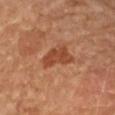Clinical impression:
Captured during whole-body skin photography for melanoma surveillance; the lesion was not biopsied.
Background:
An algorithmic analysis of the crop reported a border-irregularity rating of about 4/10, internal color variation of about 2 on a 0–10 scale, and radial color variation of about 0.5. Cropped from a whole-body photographic skin survey; the tile spans about 15 mm. Located on the right forearm. The patient is a female aged 68–72.